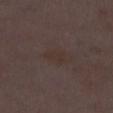* biopsy status · total-body-photography surveillance lesion; no biopsy
* anatomic site · the leg
* tile lighting · white-light illumination
* image-analysis metrics · a footprint of about 3 mm², a shape eccentricity near 0.85, and a shape-asymmetry score of about 0.4 (0 = symmetric); a mean CIELAB color near L≈31 a*≈12 b*≈18, roughly 3 lightness units darker than nearby skin, and a normalized lesion–skin contrast near 5.5; a border-irregularity index near 4/10, a within-lesion color-variation index near 0/10, and radial color variation of about 0
* diameter · ~2.5 mm (longest diameter)
* image · total-body-photography crop, ~15 mm field of view
* patient · female, aged around 30